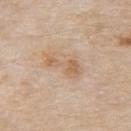  biopsy_status: not biopsied; imaged during a skin examination
  patient:
    sex: male
    age_approx: 85+
  site: back
  image:
    source: total-body photography crop
    field_of_view_mm: 15